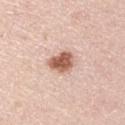A lesion tile, about 15 mm wide, cut from a 3D total-body photograph. Located on the left upper arm. The lesion-visualizer software estimated a mean CIELAB color near L≈60 a*≈22 b*≈29 and a lesion-to-skin contrast of about 10.5 (normalized; higher = more distinct). The software also gave a nevus-likeness score of about 100/100 and lesion-presence confidence of about 100/100. Approximately 3.5 mm at its widest. A male patient, aged approximately 45. Imaged with white-light lighting.